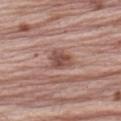Impression: Part of a total-body skin-imaging series; this lesion was reviewed on a skin check and was not flagged for biopsy. Image and clinical context: The lesion-visualizer software estimated an automated nevus-likeness rating near 5 out of 100. The subject is a male about 65 years old. Cropped from a total-body skin-imaging series; the visible field is about 15 mm. Imaged with white-light lighting. The lesion's longest dimension is about 3 mm. Located on the left upper arm.